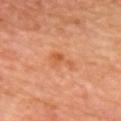Part of a total-body skin-imaging series; this lesion was reviewed on a skin check and was not flagged for biopsy. Captured under cross-polarized illumination. A close-up tile cropped from a whole-body skin photograph, about 15 mm across. Automated tile analysis of the lesion measured a lesion-detection confidence of about 100/100. A male subject approximately 60 years of age. The lesion is on the upper back.